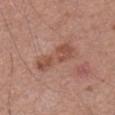Notes:
- notes · catalogued during a skin exam; not biopsied
- patient · male, aged 53–57
- tile lighting · white-light
- image · total-body-photography crop, ~15 mm field of view
- size · ~6 mm (longest diameter)
- anatomic site · the right upper arm